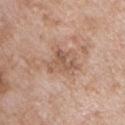workup: imaged on a skin check; not biopsied
body site: the chest
patient: male, roughly 65 years of age
image-analysis metrics: a lesion area of about 6.5 mm² and a symmetry-axis asymmetry near 0.55; a classifier nevus-likeness of about 0/100 and a detector confidence of about 100 out of 100 that the crop contains a lesion
tile lighting: white-light illumination
lesion diameter: ~3.5 mm (longest diameter)
acquisition: 15 mm crop, total-body photography